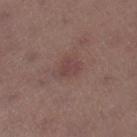Impression: Part of a total-body skin-imaging series; this lesion was reviewed on a skin check and was not flagged for biopsy. Context: This image is a 15 mm lesion crop taken from a total-body photograph. An algorithmic analysis of the crop reported an area of roughly 5.5 mm², an eccentricity of roughly 0.7, and two-axis asymmetry of about 0.35. The analysis additionally found about 6 CIELAB-L* units darker than the surrounding skin and a normalized lesion–skin contrast near 5. The software also gave internal color variation of about 3 on a 0–10 scale. The software also gave a classifier nevus-likeness of about 5/100 and lesion-presence confidence of about 100/100. A female patient in their mid- to late 50s. Imaged with white-light lighting. The lesion is located on the right lower leg. Measured at roughly 3 mm in maximum diameter.